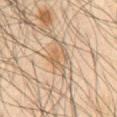Assessment: This lesion was catalogued during total-body skin photography and was not selected for biopsy. Context: A male patient, aged 63 to 67. The lesion is located on the abdomen. A lesion tile, about 15 mm wide, cut from a 3D total-body photograph.